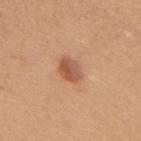biopsy_status: not biopsied; imaged during a skin examination
automated_metrics:
  area_mm2_approx: 5.5
  eccentricity: 0.75
  cielab_L: 55
  cielab_a: 24
  cielab_b: 33
  vs_skin_darker_L: 11.0
  vs_skin_contrast_norm: 7.5
  border_irregularity_0_10: 1.5
  color_variation_0_10: 4.0
  peripheral_color_asymmetry: 1.5
  lesion_detection_confidence_0_100: 100
lesion_size:
  long_diameter_mm_approx: 3.0
image:
  source: total-body photography crop
  field_of_view_mm: 15
site: upper back
patient:
  sex: female
  age_approx: 55
lighting: white-light